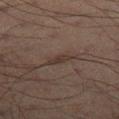Case summary:
• follow-up — imaged on a skin check; not biopsied
• lesion diameter — about 2.5 mm
• image — 15 mm crop, total-body photography
• tile lighting — cross-polarized
• patient — male, aged around 70
• site — the leg